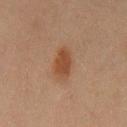Captured during whole-body skin photography for melanoma surveillance; the lesion was not biopsied. Automated tile analysis of the lesion measured a footprint of about 8 mm², an eccentricity of roughly 0.85, and a shape-asymmetry score of about 0.2 (0 = symmetric). It also reported an average lesion color of about L≈38 a*≈18 b*≈28 (CIELAB) and roughly 8 lightness units darker than nearby skin. A male subject about 45 years old. About 4.5 mm across. The lesion is located on the chest. A region of skin cropped from a whole-body photographic capture, roughly 15 mm wide.